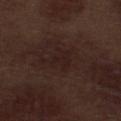The lesion was photographed on a routine skin check and not biopsied; there is no pathology result. Automated image analysis of the tile measured internal color variation of about 2 on a 0–10 scale. And it measured a nevus-likeness score of about 0/100 and lesion-presence confidence of about 100/100. Longest diameter approximately 3 mm. The lesion is on the leg. A 15 mm close-up extracted from a 3D total-body photography capture. A male subject aged approximately 70. Imaged with white-light lighting.